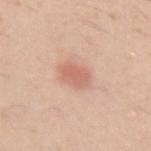* workup: total-body-photography surveillance lesion; no biopsy
* imaging modality: 15 mm crop, total-body photography
* size: ≈3.5 mm
* subject: male, aged 28 to 32
* lighting: white-light illumination
* location: the left upper arm